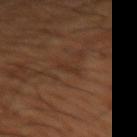biopsy status — no biopsy performed (imaged during a skin exam)
image source — ~15 mm crop, total-body skin-cancer survey
subject — male, aged approximately 65
site — the left thigh